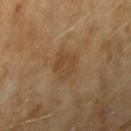Located on the arm.
Imaged with cross-polarized lighting.
Longest diameter approximately 3.5 mm.
A region of skin cropped from a whole-body photographic capture, roughly 15 mm wide.
The subject is a female aged around 60.